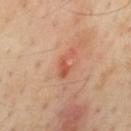Assessment: This lesion was catalogued during total-body skin photography and was not selected for biopsy. Image and clinical context: On the mid back. A male subject aged around 55. A close-up tile cropped from a whole-body skin photograph, about 15 mm across. This is a cross-polarized tile. Longest diameter approximately 3.5 mm.